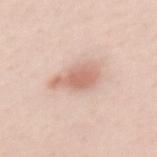The lesion was photographed on a routine skin check and not biopsied; there is no pathology result.
Captured under white-light illumination.
A female subject aged approximately 45.
An algorithmic analysis of the crop reported a color-variation rating of about 2.5/10 and peripheral color asymmetry of about 0.5.
Located on the mid back.
Measured at roughly 4.5 mm in maximum diameter.
A 15 mm crop from a total-body photograph taken for skin-cancer surveillance.